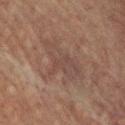- workup — no biopsy performed (imaged during a skin exam)
- site — the front of the torso
- diameter — ≈7 mm
- TBP lesion metrics — border irregularity of about 7.5 on a 0–10 scale and a peripheral color-asymmetry measure near 1; an automated nevus-likeness rating near 0 out of 100 and a lesion-detection confidence of about 80/100
- patient — male, aged approximately 60
- imaging modality — 15 mm crop, total-body photography
- illumination — cross-polarized illumination